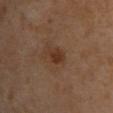No biopsy was performed on this lesion — it was imaged during a full skin examination and was not determined to be concerning. A female subject aged approximately 70. The tile uses cross-polarized illumination. Located on the upper back. An algorithmic analysis of the crop reported a footprint of about 4.5 mm². The analysis additionally found a classifier nevus-likeness of about 80/100 and lesion-presence confidence of about 100/100. A roughly 15 mm field-of-view crop from a total-body skin photograph. Longest diameter approximately 3 mm.